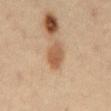This lesion was catalogued during total-body skin photography and was not selected for biopsy. This is a cross-polarized tile. A 15 mm close-up tile from a total-body photography series done for melanoma screening. A male patient aged approximately 40. Longest diameter approximately 4 mm. On the abdomen.